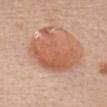Part of a total-body skin-imaging series; this lesion was reviewed on a skin check and was not flagged for biopsy. From the head or neck. The subject is a female approximately 40 years of age. The lesion-visualizer software estimated an area of roughly 27 mm², an eccentricity of roughly 0.7, and a shape-asymmetry score of about 0.4 (0 = symmetric). And it measured internal color variation of about 4.5 on a 0–10 scale and peripheral color asymmetry of about 1.5. The software also gave a nevus-likeness score of about 100/100. A 15 mm crop from a total-body photograph taken for skin-cancer surveillance. The tile uses white-light illumination.